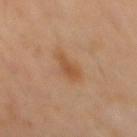Clinical impression: Part of a total-body skin-imaging series; this lesion was reviewed on a skin check and was not flagged for biopsy. Context: A male patient, aged 68–72. This is a cross-polarized tile. The total-body-photography lesion software estimated a lesion area of about 4.5 mm², an eccentricity of roughly 0.85, and two-axis asymmetry of about 0.3. It also reported a mean CIELAB color near L≈50 a*≈21 b*≈35 and a normalized lesion–skin contrast near 7. A close-up tile cropped from a whole-body skin photograph, about 15 mm across. Approximately 3 mm at its widest. On the mid back.